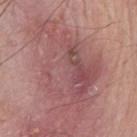Recorded during total-body skin imaging; not selected for excision or biopsy.
A male subject, in their 80s.
Imaged with white-light lighting.
Cropped from a total-body skin-imaging series; the visible field is about 15 mm.
The lesion-visualizer software estimated a lesion area of about 42 mm² and a shape-asymmetry score of about 0.45 (0 = symmetric). The analysis additionally found a border-irregularity index near 8/10 and internal color variation of about 6.5 on a 0–10 scale. It also reported an automated nevus-likeness rating near 0 out of 100 and a lesion-detection confidence of about 85/100.
On the front of the torso.